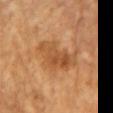| key | value |
|---|---|
| notes | imaged on a skin check; not biopsied |
| tile lighting | cross-polarized |
| anatomic site | the chest |
| acquisition | ~15 mm crop, total-body skin-cancer survey |
| subject | female, approximately 70 years of age |
| lesion size | ≈5.5 mm |
| image-analysis metrics | an outline eccentricity of about 0.75 (0 = round, 1 = elongated) and a shape-asymmetry score of about 0.35 (0 = symmetric); a lesion-detection confidence of about 100/100 |